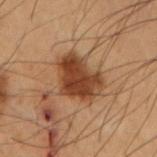Part of a total-body skin-imaging series; this lesion was reviewed on a skin check and was not flagged for biopsy.
This is a cross-polarized tile.
A male patient, in their mid-50s.
About 4.5 mm across.
A region of skin cropped from a whole-body photographic capture, roughly 15 mm wide.
From the left forearm.